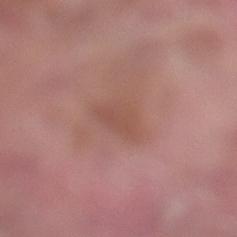No biopsy was performed on this lesion — it was imaged during a full skin examination and was not determined to be concerning.
Approximately 3.5 mm at its widest.
Located on the left lower leg.
A male subject, roughly 40 years of age.
The total-body-photography lesion software estimated a footprint of about 6 mm², an eccentricity of roughly 0.8, and a shape-asymmetry score of about 0.35 (0 = symmetric). The analysis additionally found an average lesion color of about L≈51 a*≈22 b*≈26 (CIELAB), roughly 6 lightness units darker than nearby skin, and a lesion-to-skin contrast of about 5 (normalized; higher = more distinct). The software also gave a nevus-likeness score of about 0/100 and a lesion-detection confidence of about 100/100.
A roughly 15 mm field-of-view crop from a total-body skin photograph.
Imaged with white-light lighting.